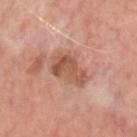notes = total-body-photography surveillance lesion; no biopsy | lighting = cross-polarized illumination | image = total-body-photography crop, ~15 mm field of view | subject = male, aged 63–67 | body site = the head or neck.